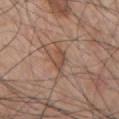Impression:
Part of a total-body skin-imaging series; this lesion was reviewed on a skin check and was not flagged for biopsy.
Context:
A region of skin cropped from a whole-body photographic capture, roughly 15 mm wide. A male subject, in their 70s. Automated image analysis of the tile measured a lesion area of about 3.5 mm², an outline eccentricity of about 0.9 (0 = round, 1 = elongated), and a symmetry-axis asymmetry near 0.3. The analysis additionally found an average lesion color of about L≈49 a*≈19 b*≈28 (CIELAB) and about 8 CIELAB-L* units darker than the surrounding skin. It also reported a border-irregularity index near 3.5/10, a color-variation rating of about 1/10, and peripheral color asymmetry of about 0.5. The lesion is located on the chest. Imaged with white-light lighting.